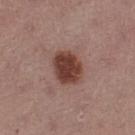notes: catalogued during a skin exam; not biopsied
image: ~15 mm crop, total-body skin-cancer survey
patient: female, aged 53–57
anatomic site: the left thigh
TBP lesion metrics: an average lesion color of about L≈40 a*≈22 b*≈24 (CIELAB), roughly 14 lightness units darker than nearby skin, and a lesion-to-skin contrast of about 11.5 (normalized; higher = more distinct); a classifier nevus-likeness of about 95/100 and lesion-presence confidence of about 100/100